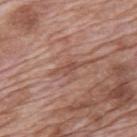acquisition: ~15 mm crop, total-body skin-cancer survey | automated lesion analysis: a mean CIELAB color near L≈50 a*≈22 b*≈26, about 8 CIELAB-L* units darker than the surrounding skin, and a normalized border contrast of about 6 | subject: male, in their 70s | tile lighting: white-light | anatomic site: the mid back | lesion diameter: about 3 mm.